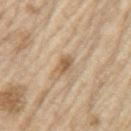Captured during whole-body skin photography for melanoma surveillance; the lesion was not biopsied. On the left upper arm. Approximately 3 mm at its widest. Cropped from a whole-body photographic skin survey; the tile spans about 15 mm. Captured under white-light illumination. The patient is a male roughly 70 years of age.